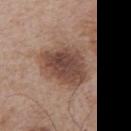The lesion was tiled from a total-body skin photograph and was not biopsied. A male patient aged around 65. The lesion is on the front of the torso. A lesion tile, about 15 mm wide, cut from a 3D total-body photograph.